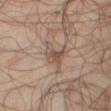<tbp_lesion>
<biopsy_status>not biopsied; imaged during a skin examination</biopsy_status>
<automated_metrics>
  <cielab_L>48</cielab_L>
  <cielab_a>15</cielab_a>
  <cielab_b>23</cielab_b>
  <vs_skin_darker_L>8.0</vs_skin_darker_L>
  <vs_skin_contrast_norm>6.5</vs_skin_contrast_norm>
  <border_irregularity_0_10>5.5</border_irregularity_0_10>
  <color_variation_0_10>3.5</color_variation_0_10>
  <peripheral_color_asymmetry>1.0</peripheral_color_asymmetry>
</automated_metrics>
<lesion_size>
  <long_diameter_mm_approx>4.0</long_diameter_mm_approx>
</lesion_size>
<lighting>cross-polarized</lighting>
<site>right thigh</site>
<image>
  <source>total-body photography crop</source>
  <field_of_view_mm>15</field_of_view_mm>
</image>
<patient>
  <sex>male</sex>
  <age_approx>65</age_approx>
</patient>
</tbp_lesion>